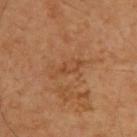The lesion was photographed on a routine skin check and not biopsied; there is no pathology result. A 15 mm close-up tile from a total-body photography series done for melanoma screening. Imaged with cross-polarized lighting. A male subject approximately 60 years of age. An algorithmic analysis of the crop reported a lesion–skin lightness drop of about 6 and a normalized lesion–skin contrast near 5. And it measured a border-irregularity index near 5/10, a within-lesion color-variation index near 2.5/10, and radial color variation of about 1. It also reported a classifier nevus-likeness of about 0/100 and lesion-presence confidence of about 100/100. From the upper back. Longest diameter approximately 4 mm.